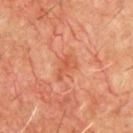The lesion is on the chest.
Longest diameter approximately 3 mm.
The subject is a male aged 58 to 62.
A region of skin cropped from a whole-body photographic capture, roughly 15 mm wide.
The total-body-photography lesion software estimated a mean CIELAB color near L≈53 a*≈29 b*≈37 and a normalized lesion–skin contrast near 5.5.
Imaged with cross-polarized lighting.